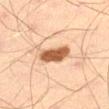Q: Was a biopsy performed?
A: total-body-photography surveillance lesion; no biopsy
Q: What are the patient's age and sex?
A: male, aged 43–47
Q: Lesion location?
A: the right thigh
Q: What is the imaging modality?
A: ~15 mm tile from a whole-body skin photo
Q: How was the tile lit?
A: cross-polarized